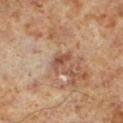biopsy status: imaged on a skin check; not biopsied
patient: male, roughly 60 years of age
body site: the left lower leg
image-analysis metrics: roughly 9 lightness units darker than nearby skin and a lesion-to-skin contrast of about 7 (normalized; higher = more distinct); an automated nevus-likeness rating near 0 out of 100 and lesion-presence confidence of about 95/100
image: ~15 mm tile from a whole-body skin photo
lesion diameter: ≈2.5 mm